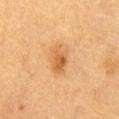Q: Was a biopsy performed?
A: imaged on a skin check; not biopsied
Q: How was this image acquired?
A: ~15 mm tile from a whole-body skin photo
Q: Patient demographics?
A: male, roughly 85 years of age or older
Q: Lesion size?
A: ≈4 mm
Q: Lesion location?
A: the front of the torso
Q: Illumination type?
A: cross-polarized illumination